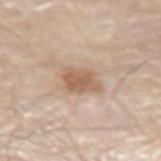workup: no biopsy performed (imaged during a skin exam)
lesion diameter: about 4 mm
image-analysis metrics: a lesion area of about 9 mm² and two-axis asymmetry of about 0.25; a lesion color around L≈60 a*≈17 b*≈31 in CIELAB and a lesion-to-skin contrast of about 7.5 (normalized; higher = more distinct); a border-irregularity index near 2.5/10, a color-variation rating of about 3/10, and radial color variation of about 1; a nevus-likeness score of about 85/100 and a lesion-detection confidence of about 100/100
image: 15 mm crop, total-body photography
subject: male, aged approximately 80
lighting: white-light
location: the left forearm